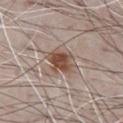Assessment: Captured during whole-body skin photography for melanoma surveillance; the lesion was not biopsied. Image and clinical context: The recorded lesion diameter is about 4 mm. A male patient, aged around 70. On the chest. Cropped from a total-body skin-imaging series; the visible field is about 15 mm. Imaged with white-light lighting.